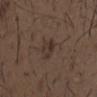Part of a total-body skin-imaging series; this lesion was reviewed on a skin check and was not flagged for biopsy. From the chest. The total-body-photography lesion software estimated a lesion color around L≈32 a*≈13 b*≈21 in CIELAB, about 6 CIELAB-L* units darker than the surrounding skin, and a lesion-to-skin contrast of about 6.5 (normalized; higher = more distinct). The software also gave a within-lesion color-variation index near 3.5/10. The lesion's longest dimension is about 3 mm. A 15 mm crop from a total-body photograph taken for skin-cancer surveillance. The subject is a male approximately 50 years of age.